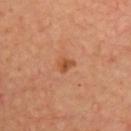- diameter: ≈2 mm
- subject: male, aged approximately 65
- site: the chest
- image source: 15 mm crop, total-body photography